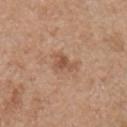{
  "biopsy_status": "not biopsied; imaged during a skin examination",
  "image": {
    "source": "total-body photography crop",
    "field_of_view_mm": 15
  },
  "patient": {
    "sex": "male",
    "age_approx": 55
  },
  "site": "front of the torso"
}